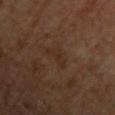The lesion was photographed on a routine skin check and not biopsied; there is no pathology result. An algorithmic analysis of the crop reported a lesion color around L≈27 a*≈16 b*≈25 in CIELAB, roughly 4 lightness units darker than nearby skin, and a normalized border contrast of about 5.5. The software also gave border irregularity of about 6.5 on a 0–10 scale, internal color variation of about 0 on a 0–10 scale, and peripheral color asymmetry of about 0. It also reported an automated nevus-likeness rating near 0 out of 100 and lesion-presence confidence of about 100/100. From the upper back. A female patient about 65 years old. Cropped from a total-body skin-imaging series; the visible field is about 15 mm.